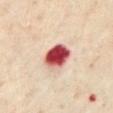Recorded during total-body skin imaging; not selected for excision or biopsy. A lesion tile, about 15 mm wide, cut from a 3D total-body photograph. About 4 mm across. The lesion-visualizer software estimated a border-irregularity rating of about 1/10, internal color variation of about 9 on a 0–10 scale, and radial color variation of about 2.5. The lesion is located on the chest. The patient is a female approximately 60 years of age.